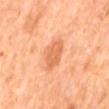Imaged during a routine full-body skin examination; the lesion was not biopsied and no histopathology is available.
An algorithmic analysis of the crop reported two-axis asymmetry of about 0.2.
A 15 mm close-up extracted from a 3D total-body photography capture.
Located on the mid back.
Captured under cross-polarized illumination.
The subject is a male approximately 65 years of age.